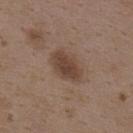Captured during whole-body skin photography for melanoma surveillance; the lesion was not biopsied. A female subject, aged 33–37. Longest diameter approximately 4.5 mm. A close-up tile cropped from a whole-body skin photograph, about 15 mm across. From the upper back.